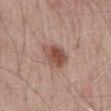Impression:
Captured during whole-body skin photography for melanoma surveillance; the lesion was not biopsied.
Image and clinical context:
This is a white-light tile. The lesion is located on the abdomen. The lesion's longest dimension is about 3.5 mm. Automated tile analysis of the lesion measured a lesion area of about 8 mm², a shape eccentricity near 0.55, and two-axis asymmetry of about 0.25. The analysis additionally found a border-irregularity rating of about 2.5/10, internal color variation of about 5 on a 0–10 scale, and radial color variation of about 1.5. It also reported lesion-presence confidence of about 100/100. The subject is a male in their mid- to late 60s. A 15 mm crop from a total-body photograph taken for skin-cancer surveillance.